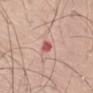workup: catalogued during a skin exam; not biopsied
anatomic site: the right upper arm
imaging modality: ~15 mm tile from a whole-body skin photo
lighting: white-light
subject: male, aged approximately 50
lesion size: about 2.5 mm